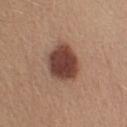This lesion was catalogued during total-body skin photography and was not selected for biopsy.